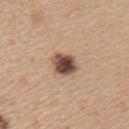Findings:
• tile lighting — white-light illumination
• lesion size — ~3.5 mm (longest diameter)
• anatomic site — the upper back
• subject — female, approximately 45 years of age
• imaging modality — 15 mm crop, total-body photography